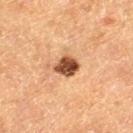biopsy status — no biopsy performed (imaged during a skin exam)
subject — male, aged around 75
location — the leg
automated lesion analysis — an area of roughly 6.5 mm², an eccentricity of roughly 0.45, and two-axis asymmetry of about 0.25; a lesion color around L≈40 a*≈20 b*≈30 in CIELAB and roughly 17 lightness units darker than nearby skin; a border-irregularity rating of about 2/10 and a peripheral color-asymmetry measure near 1.5; a classifier nevus-likeness of about 90/100 and a detector confidence of about 100 out of 100 that the crop contains a lesion
tile lighting — cross-polarized
image — 15 mm crop, total-body photography
diameter — ~3 mm (longest diameter)